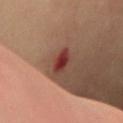No biopsy was performed on this lesion — it was imaged during a full skin examination and was not determined to be concerning. Measured at roughly 3 mm in maximum diameter. The lesion is on the mid back. A 15 mm crop from a total-body photograph taken for skin-cancer surveillance. The subject is a female aged 58 to 62.